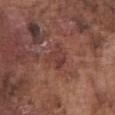This lesion was catalogued during total-body skin photography and was not selected for biopsy. Automated tile analysis of the lesion measured a mean CIELAB color near L≈39 a*≈23 b*≈23, roughly 6 lightness units darker than nearby skin, and a lesion-to-skin contrast of about 6 (normalized; higher = more distinct). Located on the front of the torso. A male subject, approximately 75 years of age. A roughly 15 mm field-of-view crop from a total-body skin photograph. The lesion's longest dimension is about 3 mm. This is a white-light tile.